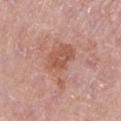  biopsy_status: not biopsied; imaged during a skin examination
  lesion_size:
    long_diameter_mm_approx: 5.5
  site: left forearm
  image:
    source: total-body photography crop
    field_of_view_mm: 15
  patient:
    sex: female
    age_approx: 65